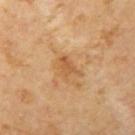Impression: The lesion was tiled from a total-body skin photograph and was not biopsied. Background: The patient is a female approximately 45 years of age. From the left upper arm. An algorithmic analysis of the crop reported a lesion area of about 3.5 mm², a shape eccentricity near 0.75, and a symmetry-axis asymmetry near 0.4. It also reported border irregularity of about 4 on a 0–10 scale, a within-lesion color-variation index near 1.5/10, and radial color variation of about 0.5. The analysis additionally found a classifier nevus-likeness of about 0/100 and a detector confidence of about 100 out of 100 that the crop contains a lesion. A 15 mm close-up tile from a total-body photography series done for melanoma screening. Approximately 3 mm at its widest.